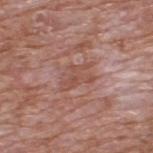workup: no biopsy performed (imaged during a skin exam) | subject: male, aged 58 to 62 | body site: the upper back | imaging modality: ~15 mm crop, total-body skin-cancer survey | illumination: white-light illumination | automated metrics: a mean CIELAB color near L≈51 a*≈23 b*≈26, about 6 CIELAB-L* units darker than the surrounding skin, and a lesion-to-skin contrast of about 5 (normalized; higher = more distinct); a detector confidence of about 90 out of 100 that the crop contains a lesion.